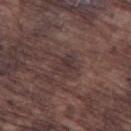The lesion was photographed on a routine skin check and not biopsied; there is no pathology result. Automated image analysis of the tile measured a lesion–skin lightness drop of about 5. Captured under white-light illumination. The lesion is on the left thigh. The recorded lesion diameter is about 3 mm. A male subject, aged 73–77. A lesion tile, about 15 mm wide, cut from a 3D total-body photograph.